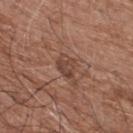workup: total-body-photography surveillance lesion; no biopsy
image source: 15 mm crop, total-body photography
site: the upper back
subject: male, approximately 75 years of age